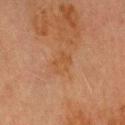Clinical impression: Imaged during a routine full-body skin examination; the lesion was not biopsied and no histopathology is available. Image and clinical context: This image is a 15 mm lesion crop taken from a total-body photograph. Captured under cross-polarized illumination. The patient is a male about 70 years old. On the head or neck. Measured at roughly 3 mm in maximum diameter. The lesion-visualizer software estimated an eccentricity of roughly 0.8 and a symmetry-axis asymmetry near 0.35. The software also gave an average lesion color of about L≈42 a*≈20 b*≈32 (CIELAB), about 4 CIELAB-L* units darker than the surrounding skin, and a normalized border contrast of about 4.5. The analysis additionally found border irregularity of about 4 on a 0–10 scale, internal color variation of about 2.5 on a 0–10 scale, and a peripheral color-asymmetry measure near 1. It also reported a classifier nevus-likeness of about 0/100 and a lesion-detection confidence of about 100/100.